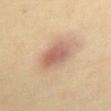notes: imaged on a skin check; not biopsied
anatomic site: the abdomen
lighting: cross-polarized illumination
subject: female, approximately 65 years of age
lesion diameter: ~5 mm (longest diameter)
image: 15 mm crop, total-body photography
TBP lesion metrics: a shape eccentricity near 0.85; roughly 12 lightness units darker than nearby skin and a lesion-to-skin contrast of about 7.5 (normalized; higher = more distinct); border irregularity of about 1.5 on a 0–10 scale, internal color variation of about 5 on a 0–10 scale, and peripheral color asymmetry of about 1.5